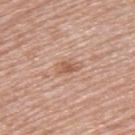No biopsy was performed on this lesion — it was imaged during a full skin examination and was not determined to be concerning. The recorded lesion diameter is about 3 mm. A female patient, in their 50s. An algorithmic analysis of the crop reported a border-irregularity index near 3.5/10. Imaged with white-light lighting. On the back. Cropped from a whole-body photographic skin survey; the tile spans about 15 mm.